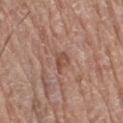Q: Was a biopsy performed?
A: no biopsy performed (imaged during a skin exam)
Q: What is the lesion's diameter?
A: ~2.5 mm (longest diameter)
Q: Patient demographics?
A: female, aged approximately 80
Q: How was this image acquired?
A: ~15 mm tile from a whole-body skin photo
Q: Lesion location?
A: the right thigh
Q: How was the tile lit?
A: white-light illumination